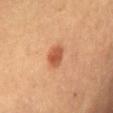{"site": "chest", "image": {"source": "total-body photography crop", "field_of_view_mm": 15}, "patient": {"sex": "female", "age_approx": 60}, "automated_metrics": {"area_mm2_approx": 5.0, "eccentricity": 0.8, "shape_asymmetry": 0.25}, "lighting": "cross-polarized", "lesion_size": {"long_diameter_mm_approx": 3.0}}